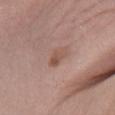Findings:
– biopsy status — imaged on a skin check; not biopsied
– image-analysis metrics — a lesion area of about 4 mm² and two-axis asymmetry of about 0.35; an average lesion color of about L≈52 a*≈20 b*≈26 (CIELAB), roughly 8 lightness units darker than nearby skin, and a normalized border contrast of about 6; a border-irregularity rating of about 3.5/10, internal color variation of about 3 on a 0–10 scale, and radial color variation of about 1; a nevus-likeness score of about 10/100
– tile lighting — white-light
– imaging modality — ~15 mm crop, total-body skin-cancer survey
– patient — female, aged approximately 40
– body site — the right lower leg
– size — about 3 mm